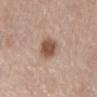Findings:
* notes · imaged on a skin check; not biopsied
* body site · the front of the torso
* image-analysis metrics · a footprint of about 8 mm², an outline eccentricity of about 0.65 (0 = round, 1 = elongated), and two-axis asymmetry of about 0.2; a lesion–skin lightness drop of about 13 and a normalized border contrast of about 9.5; a border-irregularity index near 2/10, a color-variation rating of about 3/10, and a peripheral color-asymmetry measure near 1; a nevus-likeness score of about 95/100 and a lesion-detection confidence of about 100/100
* lesion diameter · ~3.5 mm (longest diameter)
* subject · female, aged around 65
* illumination · white-light
* imaging modality · 15 mm crop, total-body photography